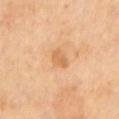Q: Was this lesion biopsied?
A: imaged on a skin check; not biopsied
Q: Automated lesion metrics?
A: a lesion color around L≈63 a*≈22 b*≈41 in CIELAB and a normalized border contrast of about 6; an automated nevus-likeness rating near 5 out of 100 and lesion-presence confidence of about 100/100
Q: What kind of image is this?
A: ~15 mm tile from a whole-body skin photo
Q: What are the patient's age and sex?
A: male, in their 70s
Q: Lesion location?
A: the left upper arm
Q: What lighting was used for the tile?
A: cross-polarized